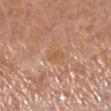biopsy_status: not biopsied; imaged during a skin examination
automated_metrics:
  area_mm2_approx: 4.0
  shape_asymmetry: 0.2
  vs_skin_darker_L: 5.0
  vs_skin_contrast_norm: 5.0
  color_variation_0_10: 1.0
  peripheral_color_asymmetry: 0.5
patient:
  sex: female
  age_approx: 55
lesion_size:
  long_diameter_mm_approx: 2.5
lighting: white-light
site: right lower leg
image:
  source: total-body photography crop
  field_of_view_mm: 15